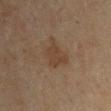No biopsy was performed on this lesion — it was imaged during a full skin examination and was not determined to be concerning. The recorded lesion diameter is about 3.5 mm. A male patient, aged 43 to 47. On the left upper arm. Imaged with cross-polarized lighting. A close-up tile cropped from a whole-body skin photograph, about 15 mm across.